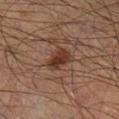Notes:
- image-analysis metrics: an area of roughly 7.5 mm², an outline eccentricity of about 0.7 (0 = round, 1 = elongated), and two-axis asymmetry of about 0.3; a lesion color around L≈27 a*≈16 b*≈21 in CIELAB and a normalized lesion–skin contrast near 8.5; a border-irregularity rating of about 3/10, a color-variation rating of about 3/10, and radial color variation of about 1; an automated nevus-likeness rating near 90 out of 100 and a detector confidence of about 100 out of 100 that the crop contains a lesion
- subject: male, in their 50s
- illumination: cross-polarized
- site: the right lower leg
- image source: ~15 mm tile from a whole-body skin photo